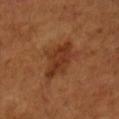Part of a total-body skin-imaging series; this lesion was reviewed on a skin check and was not flagged for biopsy. This is a cross-polarized tile. The patient is a female in their mid-50s. A 15 mm close-up tile from a total-body photography series done for melanoma screening. The lesion is on the right forearm.